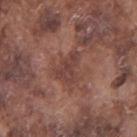Assessment: This lesion was catalogued during total-body skin photography and was not selected for biopsy. Background: Automated tile analysis of the lesion measured an average lesion color of about L≈41 a*≈21 b*≈23 (CIELAB), roughly 7 lightness units darker than nearby skin, and a lesion-to-skin contrast of about 6.5 (normalized; higher = more distinct). The software also gave border irregularity of about 5 on a 0–10 scale, a color-variation rating of about 2/10, and peripheral color asymmetry of about 1. The software also gave a nevus-likeness score of about 0/100 and a lesion-detection confidence of about 70/100. A lesion tile, about 15 mm wide, cut from a 3D total-body photograph. This is a white-light tile. The subject is a male about 75 years old. Approximately 3.5 mm at its widest. Located on the right upper arm.